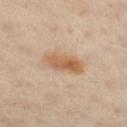The lesion was photographed on a routine skin check and not biopsied; there is no pathology result. The subject is a female aged approximately 40. Located on the leg. Cropped from a total-body skin-imaging series; the visible field is about 15 mm.